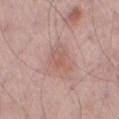Clinical summary:
The recorded lesion diameter is about 3.5 mm. Automated image analysis of the tile measured a lesion–skin lightness drop of about 7 and a lesion-to-skin contrast of about 5 (normalized; higher = more distinct). The analysis additionally found border irregularity of about 5 on a 0–10 scale. The software also gave a classifier nevus-likeness of about 10/100. A 15 mm close-up extracted from a 3D total-body photography capture. From the left thigh. The subject is a male about 55 years old.